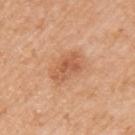This lesion was catalogued during total-body skin photography and was not selected for biopsy. A female patient in their mid-50s. On the left upper arm. Cropped from a total-body skin-imaging series; the visible field is about 15 mm.